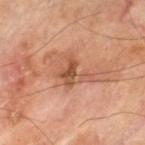| field | value |
|---|---|
| follow-up | no biopsy performed (imaged during a skin exam) |
| body site | the left thigh |
| subject | male, aged approximately 70 |
| lighting | cross-polarized illumination |
| imaging modality | ~15 mm crop, total-body skin-cancer survey |
| lesion diameter | about 4 mm |